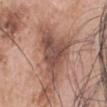Q: Was a biopsy performed?
A: imaged on a skin check; not biopsied
Q: Lesion size?
A: ~7 mm (longest diameter)
Q: Lesion location?
A: the head or neck
Q: How was the tile lit?
A: white-light illumination
Q: What is the imaging modality?
A: 15 mm crop, total-body photography
Q: Who is the patient?
A: male, aged 63 to 67